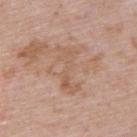biopsy status: no biopsy performed (imaged during a skin exam)
TBP lesion metrics: an area of roughly 8.5 mm² and an outline eccentricity of about 0.95 (0 = round, 1 = elongated); a peripheral color-asymmetry measure near 0.5; a classifier nevus-likeness of about 0/100 and lesion-presence confidence of about 100/100
lighting: white-light
body site: the back
acquisition: ~15 mm crop, total-body skin-cancer survey
subject: male, aged around 65